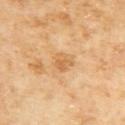Clinical impression:
This lesion was catalogued during total-body skin photography and was not selected for biopsy.
Background:
Captured under cross-polarized illumination. Approximately 2.5 mm at its widest. A female patient about 60 years old. A region of skin cropped from a whole-body photographic capture, roughly 15 mm wide. Automated tile analysis of the lesion measured an outline eccentricity of about 0.6 (0 = round, 1 = elongated) and a symmetry-axis asymmetry near 0.3. Located on the upper back.